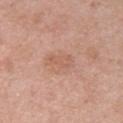The lesion was tiled from a total-body skin photograph and was not biopsied.
Automated image analysis of the tile measured a footprint of about 4 mm². And it measured an average lesion color of about L≈59 a*≈22 b*≈30 (CIELAB), roughly 6 lightness units darker than nearby skin, and a normalized lesion–skin contrast near 4.5. The software also gave peripheral color asymmetry of about 0.5. And it measured an automated nevus-likeness rating near 0 out of 100 and lesion-presence confidence of about 100/100.
From the chest.
The tile uses white-light illumination.
About 3 mm across.
A region of skin cropped from a whole-body photographic capture, roughly 15 mm wide.
The patient is a male aged approximately 55.